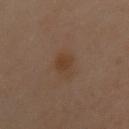Imaged during a routine full-body skin examination; the lesion was not biopsied and no histopathology is available.
The patient is a female roughly 60 years of age.
Captured under cross-polarized illumination.
The lesion is on the chest.
An algorithmic analysis of the crop reported a footprint of about 5 mm², an eccentricity of roughly 0.6, and two-axis asymmetry of about 0.25. And it measured roughly 5 lightness units darker than nearby skin. The analysis additionally found border irregularity of about 2.5 on a 0–10 scale, a within-lesion color-variation index near 1.5/10, and peripheral color asymmetry of about 0.5.
Cropped from a total-body skin-imaging series; the visible field is about 15 mm.